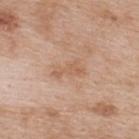Impression:
Recorded during total-body skin imaging; not selected for excision or biopsy.
Acquisition and patient details:
The patient is a female aged around 40. A close-up tile cropped from a whole-body skin photograph, about 15 mm across. The lesion is located on the upper back. Approximately 3.5 mm at its widest. This is a white-light tile. The total-body-photography lesion software estimated a lesion area of about 5 mm², an eccentricity of roughly 0.9, and two-axis asymmetry of about 0.45. The software also gave a mean CIELAB color near L≈60 a*≈19 b*≈32, about 7 CIELAB-L* units darker than the surrounding skin, and a normalized lesion–skin contrast near 5. It also reported a nevus-likeness score of about 0/100 and lesion-presence confidence of about 100/100.